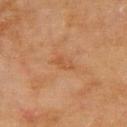Part of a total-body skin-imaging series; this lesion was reviewed on a skin check and was not flagged for biopsy. A female subject aged 78 to 82. On the upper back. Cropped from a total-body skin-imaging series; the visible field is about 15 mm.